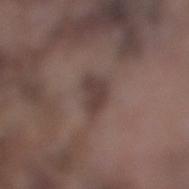workup: imaged on a skin check; not biopsied
subject: male, aged around 75
acquisition: total-body-photography crop, ~15 mm field of view
site: the leg